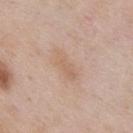<lesion>
<biopsy_status>not biopsied; imaged during a skin examination</biopsy_status>
<lighting>white-light</lighting>
<lesion_size>
  <long_diameter_mm_approx>4.0</long_diameter_mm_approx>
</lesion_size>
<automated_metrics>
  <eccentricity>0.9</eccentricity>
  <shape_asymmetry>0.25</shape_asymmetry>
  <cielab_L>62</cielab_L>
  <cielab_a>17</cielab_a>
  <cielab_b>30</cielab_b>
  <vs_skin_darker_L>6.0</vs_skin_darker_L>
  <vs_skin_contrast_norm>5.0</vs_skin_contrast_norm>
  <border_irregularity_0_10>3.5</border_irregularity_0_10>
  <color_variation_0_10>2.5</color_variation_0_10>
  <peripheral_color_asymmetry>1.0</peripheral_color_asymmetry>
  <nevus_likeness_0_100>0</nevus_likeness_0_100>
  <lesion_detection_confidence_0_100>100</lesion_detection_confidence_0_100>
</automated_metrics>
<site>chest</site>
<image>
  <source>total-body photography crop</source>
  <field_of_view_mm>15</field_of_view_mm>
</image>
<patient>
  <sex>male</sex>
  <age_approx>55</age_approx>
</patient>
</lesion>